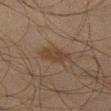Image and clinical context:
Automated tile analysis of the lesion measured an average lesion color of about L≈32 a*≈13 b*≈23 (CIELAB), about 6 CIELAB-L* units darker than the surrounding skin, and a normalized border contrast of about 6.5. It also reported border irregularity of about 2.5 on a 0–10 scale, a color-variation rating of about 2/10, and radial color variation of about 0.5. A 15 mm crop from a total-body photograph taken for skin-cancer surveillance. The subject is a male in their mid- to late 40s. Imaged with cross-polarized lighting. From the left thigh. The recorded lesion diameter is about 4 mm.